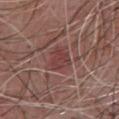Q: Was a biopsy performed?
A: imaged on a skin check; not biopsied
Q: Patient demographics?
A: male, in their mid- to late 70s
Q: Where on the body is the lesion?
A: the front of the torso
Q: How large is the lesion?
A: about 4 mm
Q: What kind of image is this?
A: ~15 mm tile from a whole-body skin photo